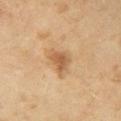biopsy_status: not biopsied; imaged during a skin examination
site: right upper arm
lesion_size:
  long_diameter_mm_approx: 3.0
image:
  source: total-body photography crop
  field_of_view_mm: 15
patient:
  sex: female
  age_approx: 60
lighting: cross-polarized